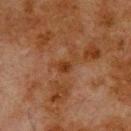{"biopsy_status": "not biopsied; imaged during a skin examination", "lighting": "cross-polarized", "patient": {"sex": "male", "age_approx": 80}, "lesion_size": {"long_diameter_mm_approx": 3.0}, "image": {"source": "total-body photography crop", "field_of_view_mm": 15}, "site": "upper back"}